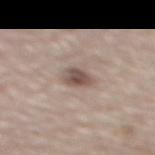Notes:
* workup: no biopsy performed (imaged during a skin exam)
* subject: male, about 75 years old
* imaging modality: ~15 mm crop, total-body skin-cancer survey
* lesion size: about 3 mm
* lighting: white-light
* automated lesion analysis: an average lesion color of about L≈52 a*≈14 b*≈21 (CIELAB), a lesion–skin lightness drop of about 12, and a normalized border contrast of about 8.5
* body site: the mid back